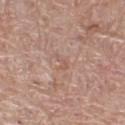Clinical impression: The lesion was photographed on a routine skin check and not biopsied; there is no pathology result. Image and clinical context: A lesion tile, about 15 mm wide, cut from a 3D total-body photograph. A female patient, aged 58 to 62. The total-body-photography lesion software estimated an area of roughly 2 mm², an outline eccentricity of about 0.9 (0 = round, 1 = elongated), and a shape-asymmetry score of about 0.55 (0 = symmetric). The analysis additionally found a mean CIELAB color near L≈57 a*≈20 b*≈27. The analysis additionally found an automated nevus-likeness rating near 0 out of 100 and lesion-presence confidence of about 95/100. The tile uses white-light illumination. From the leg.